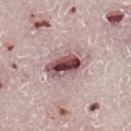The lesion was photographed on a routine skin check and not biopsied; there is no pathology result.
Imaged with white-light lighting.
The lesion is on the right lower leg.
About 5 mm across.
This image is a 15 mm lesion crop taken from a total-body photograph.
The patient is a male in their mid-60s.